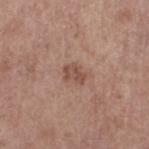notes: catalogued during a skin exam; not biopsied
lesion size: ~2.5 mm (longest diameter)
lighting: white-light
anatomic site: the left lower leg
image source: 15 mm crop, total-body photography
subject: female, in their mid- to late 60s
TBP lesion metrics: a footprint of about 3.5 mm², an outline eccentricity of about 0.75 (0 = round, 1 = elongated), and a symmetry-axis asymmetry near 0.3; border irregularity of about 3 on a 0–10 scale, a within-lesion color-variation index near 2.5/10, and a peripheral color-asymmetry measure near 1; a nevus-likeness score of about 15/100 and a lesion-detection confidence of about 100/100